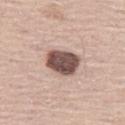Case summary:
• follow-up: total-body-photography surveillance lesion; no biopsy
• anatomic site: the left thigh
• subject: male, approximately 65 years of age
• imaging modality: 15 mm crop, total-body photography